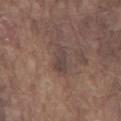The lesion was photographed on a routine skin check and not biopsied; there is no pathology result. Captured under white-light illumination. A 15 mm crop from a total-body photograph taken for skin-cancer surveillance. The subject is a male roughly 75 years of age. The lesion is on the chest. About 3.5 mm across.